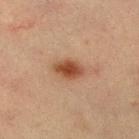workup: no biopsy performed (imaged during a skin exam) | anatomic site: the right lower leg | patient: female, aged approximately 55 | illumination: cross-polarized illumination | lesion diameter: ~3.5 mm (longest diameter) | acquisition: total-body-photography crop, ~15 mm field of view.